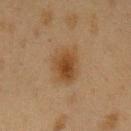Impression: The lesion was photographed on a routine skin check and not biopsied; there is no pathology result. Image and clinical context: The lesion is located on the left upper arm. A female patient, aged 38–42. This image is a 15 mm lesion crop taken from a total-body photograph.